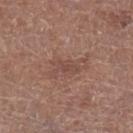Clinical impression:
The lesion was photographed on a routine skin check and not biopsied; there is no pathology result.
Acquisition and patient details:
Automated tile analysis of the lesion measured border irregularity of about 4.5 on a 0–10 scale and peripheral color asymmetry of about 0.5. The software also gave a classifier nevus-likeness of about 0/100 and a lesion-detection confidence of about 100/100. From the left lower leg. A close-up tile cropped from a whole-body skin photograph, about 15 mm across. The tile uses white-light illumination. A male subject aged 73–77. The recorded lesion diameter is about 3 mm.